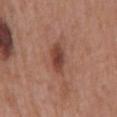| key | value |
|---|---|
| tile lighting | white-light illumination |
| location | the back |
| acquisition | 15 mm crop, total-body photography |
| patient | male, aged 68–72 |
| automated lesion analysis | a border-irregularity rating of about 2/10, a color-variation rating of about 4.5/10, and radial color variation of about 1 |
| lesion size | ~3.5 mm (longest diameter) |